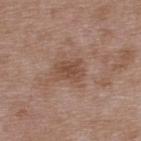Approximately 3 mm at its widest. A close-up tile cropped from a whole-body skin photograph, about 15 mm across. The subject is a male in their 50s. The lesion is located on the upper back. The tile uses white-light illumination.